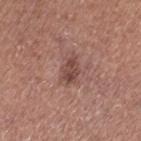Q: Was this lesion biopsied?
A: catalogued during a skin exam; not biopsied
Q: What kind of image is this?
A: ~15 mm crop, total-body skin-cancer survey
Q: Patient demographics?
A: female, aged 48–52
Q: Where on the body is the lesion?
A: the left thigh
Q: What is the lesion's diameter?
A: ~3 mm (longest diameter)
Q: Automated lesion metrics?
A: a lesion area of about 4.5 mm², a shape eccentricity near 0.8, and two-axis asymmetry of about 0.25; a border-irregularity index near 2.5/10, a color-variation rating of about 3/10, and peripheral color asymmetry of about 1; a nevus-likeness score of about 25/100 and a detector confidence of about 100 out of 100 that the crop contains a lesion